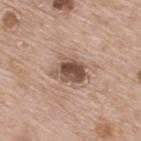Findings:
– workup · catalogued during a skin exam; not biopsied
– size · about 4.5 mm
– location · the upper back
– patient · male, about 65 years old
– image · total-body-photography crop, ~15 mm field of view
– illumination · white-light illumination